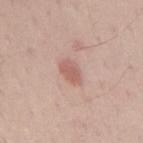notes=no biopsy performed (imaged during a skin exam); imaging modality=~15 mm crop, total-body skin-cancer survey; patient=male, aged 68–72; body site=the mid back.